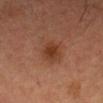Assessment:
No biopsy was performed on this lesion — it was imaged during a full skin examination and was not determined to be concerning.
Image and clinical context:
A male subject aged 33 to 37. A close-up tile cropped from a whole-body skin photograph, about 15 mm across. From the head or neck.